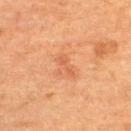Impression:
Part of a total-body skin-imaging series; this lesion was reviewed on a skin check and was not flagged for biopsy.
Clinical summary:
The recorded lesion diameter is about 3 mm. On the upper back. A female subject, approximately 70 years of age. This is a cross-polarized tile. This image is a 15 mm lesion crop taken from a total-body photograph.